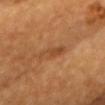<case>
<biopsy_status>not biopsied; imaged during a skin examination</biopsy_status>
<patient>
  <sex>female</sex>
  <age_approx>65</age_approx>
</patient>
<lesion_size>
  <long_diameter_mm_approx>4.5</long_diameter_mm_approx>
</lesion_size>
<image>
  <source>total-body photography crop</source>
  <field_of_view_mm>15</field_of_view_mm>
</image>
<site>chest</site>
<lighting>cross-polarized</lighting>
<automated_metrics>
  <area_mm2_approx>5.0</area_mm2_approx>
  <eccentricity>0.95</eccentricity>
  <cielab_L>47</cielab_L>
  <cielab_a>23</cielab_a>
  <cielab_b>38</cielab_b>
  <vs_skin_darker_L>7.0</vs_skin_darker_L>
  <peripheral_color_asymmetry>0.5</peripheral_color_asymmetry>
</automated_metrics>
</case>